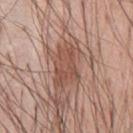{"biopsy_status": "not biopsied; imaged during a skin examination", "automated_metrics": {"vs_skin_contrast_norm": 7.0, "nevus_likeness_0_100": 45, "lesion_detection_confidence_0_100": 95}, "site": "chest", "image": {"source": "total-body photography crop", "field_of_view_mm": 15}, "lighting": "white-light", "lesion_size": {"long_diameter_mm_approx": 5.5}, "patient": {"sex": "male", "age_approx": 55}}